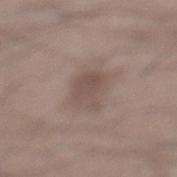{
  "biopsy_status": "not biopsied; imaged during a skin examination",
  "automated_metrics": {
    "eccentricity": 0.55,
    "shape_asymmetry": 0.3,
    "vs_skin_darker_L": 8.0,
    "vs_skin_contrast_norm": 6.0,
    "border_irregularity_0_10": 4.0,
    "color_variation_0_10": 2.0,
    "peripheral_color_asymmetry": 0.5
  },
  "site": "left lower leg",
  "lighting": "white-light",
  "patient": {
    "sex": "male",
    "age_approx": 75
  },
  "lesion_size": {
    "long_diameter_mm_approx": 3.0
  },
  "image": {
    "source": "total-body photography crop",
    "field_of_view_mm": 15
  }
}